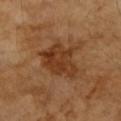Imaged during a routine full-body skin examination; the lesion was not biopsied and no histopathology is available.
An algorithmic analysis of the crop reported a mean CIELAB color near L≈38 a*≈23 b*≈35 and about 9 CIELAB-L* units darker than the surrounding skin. It also reported a border-irregularity rating of about 4/10.
The lesion is on the arm.
A female patient, about 70 years old.
The recorded lesion diameter is about 5 mm.
A 15 mm close-up tile from a total-body photography series done for melanoma screening.
Imaged with cross-polarized lighting.